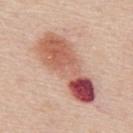location: the back
patient: male, about 60 years old
automated lesion analysis: a footprint of about 27 mm² and an outline eccentricity of about 0.95 (0 = round, 1 = elongated); a lesion color around L≈57 a*≈26 b*≈28 in CIELAB, roughly 16 lightness units darker than nearby skin, and a lesion-to-skin contrast of about 10 (normalized; higher = more distinct); a border-irregularity rating of about 4.5/10 and a peripheral color-asymmetry measure near 4.5
image: ~15 mm crop, total-body skin-cancer survey
lesion size: about 9.5 mm